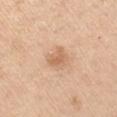About 3 mm across.
The lesion-visualizer software estimated an area of roughly 6 mm², a shape eccentricity near 0.45, and a symmetry-axis asymmetry near 0.25. It also reported an average lesion color of about L≈65 a*≈20 b*≈35 (CIELAB), about 9 CIELAB-L* units darker than the surrounding skin, and a normalized border contrast of about 6. It also reported a within-lesion color-variation index near 2/10 and radial color variation of about 1.
A 15 mm close-up tile from a total-body photography series done for melanoma screening.
The subject is a male in their 60s.
Imaged with white-light lighting.
The lesion is located on the left upper arm.